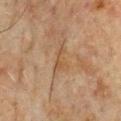Captured under cross-polarized illumination. The patient is a male roughly 65 years of age. Cropped from a whole-body photographic skin survey; the tile spans about 15 mm. From the chest. Measured at roughly 3 mm in maximum diameter.